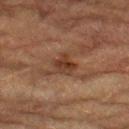| key | value |
|---|---|
| follow-up | no biopsy performed (imaged during a skin exam) |
| anatomic site | the right lower leg |
| acquisition | total-body-photography crop, ~15 mm field of view |
| patient | male, in their mid-80s or older |
| tile lighting | cross-polarized |